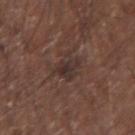Impression:
The lesion was tiled from a total-body skin photograph and was not biopsied.
Clinical summary:
A 15 mm close-up extracted from a 3D total-body photography capture. Located on the left upper arm. Automated image analysis of the tile measured an outline eccentricity of about 0.5 (0 = round, 1 = elongated) and a symmetry-axis asymmetry near 0.25. The analysis additionally found a classifier nevus-likeness of about 0/100 and a lesion-detection confidence of about 90/100. The patient is a male in their mid- to late 60s. The recorded lesion diameter is about 3.5 mm.